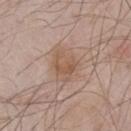biopsy status = imaged on a skin check; not biopsied
subject = male, about 55 years old
illumination = white-light illumination
lesion size = ≈3.5 mm
image = ~15 mm crop, total-body skin-cancer survey
site = the chest